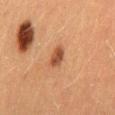illumination: cross-polarized illumination | automated lesion analysis: a shape eccentricity near 0.8 and a shape-asymmetry score of about 0.2 (0 = symmetric); a mean CIELAB color near L≈40 a*≈22 b*≈31 and about 11 CIELAB-L* units darker than the surrounding skin; a border-irregularity rating of about 2/10, internal color variation of about 3 on a 0–10 scale, and peripheral color asymmetry of about 1; a nevus-likeness score of about 90/100 and a detector confidence of about 100 out of 100 that the crop contains a lesion | patient: female, in their mid-50s | image source: 15 mm crop, total-body photography | anatomic site: the mid back | lesion size: ~3 mm (longest diameter).